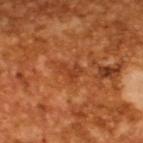| field | value |
|---|---|
| workup | imaged on a skin check; not biopsied |
| patient | male, in their mid-60s |
| lesion size | ≈2.5 mm |
| image | ~15 mm crop, total-body skin-cancer survey |